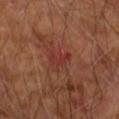Q: Was a biopsy performed?
A: imaged on a skin check; not biopsied
Q: How was this image acquired?
A: ~15 mm crop, total-body skin-cancer survey
Q: Lesion location?
A: the right upper arm
Q: What is the lesion's diameter?
A: about 3 mm
Q: Patient demographics?
A: male, in their mid- to late 60s
Q: What did automated image analysis measure?
A: a border-irregularity rating of about 5.5/10 and peripheral color asymmetry of about 0; an automated nevus-likeness rating near 0 out of 100 and a detector confidence of about 100 out of 100 that the crop contains a lesion